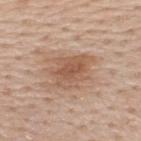A lesion tile, about 15 mm wide, cut from a 3D total-body photograph.
The lesion is on the upper back.
A male subject, in their 60s.